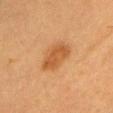Case summary:
* biopsy status: imaged on a skin check; not biopsied
* subject: female, approximately 55 years of age
* location: the head or neck
* TBP lesion metrics: a lesion area of about 9.5 mm², a shape eccentricity near 0.85, and two-axis asymmetry of about 0.2; a border-irregularity index near 2/10, a within-lesion color-variation index near 2/10, and radial color variation of about 0.5
* illumination: cross-polarized illumination
* image: ~15 mm tile from a whole-body skin photo
* size: ~4.5 mm (longest diameter)